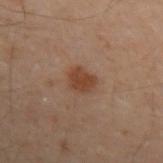Impression:
Captured during whole-body skin photography for melanoma surveillance; the lesion was not biopsied.
Background:
This is a cross-polarized tile. The lesion is located on the left forearm. A male patient, aged 48–52. A close-up tile cropped from a whole-body skin photograph, about 15 mm across.